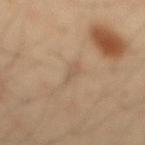Background:
A close-up tile cropped from a whole-body skin photograph, about 15 mm across. The lesion is on the mid back. An algorithmic analysis of the crop reported an area of roughly 2.5 mm² and a shape-asymmetry score of about 0.35 (0 = symmetric). It also reported a mean CIELAB color near L≈55 a*≈15 b*≈31, roughly 6 lightness units darker than nearby skin, and a lesion-to-skin contrast of about 4.5 (normalized; higher = more distinct). Imaged with cross-polarized lighting. A male subject, in their 40s. Approximately 3 mm at its widest.